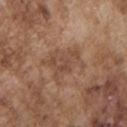Clinical impression:
No biopsy was performed on this lesion — it was imaged during a full skin examination and was not determined to be concerning.
Background:
The lesion is on the chest. The patient is a male about 75 years old. The tile uses white-light illumination. This image is a 15 mm lesion crop taken from a total-body photograph.